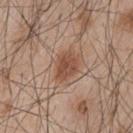Impression:
No biopsy was performed on this lesion — it was imaged during a full skin examination and was not determined to be concerning.
Image and clinical context:
A 15 mm close-up extracted from a 3D total-body photography capture. The lesion is on the mid back. A male subject, aged around 45.